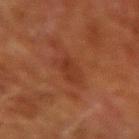<tbp_lesion>
  <biopsy_status>not biopsied; imaged during a skin examination</biopsy_status>
</tbp_lesion>